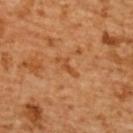Part of a total-body skin-imaging series; this lesion was reviewed on a skin check and was not flagged for biopsy.
Captured under cross-polarized illumination.
About 3 mm across.
The patient is a female aged 53 to 57.
The lesion is on the upper back.
This image is a 15 mm lesion crop taken from a total-body photograph.
Automated tile analysis of the lesion measured an area of roughly 2.5 mm² and a shape eccentricity near 0.95. The analysis additionally found an average lesion color of about L≈51 a*≈27 b*≈43 (CIELAB) and a normalized border contrast of about 6.5. It also reported border irregularity of about 7 on a 0–10 scale, a within-lesion color-variation index near 0/10, and a peripheral color-asymmetry measure near 0. And it measured an automated nevus-likeness rating near 0 out of 100 and a detector confidence of about 100 out of 100 that the crop contains a lesion.